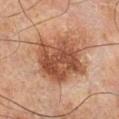Assessment:
Recorded during total-body skin imaging; not selected for excision or biopsy.
Background:
This image is a 15 mm lesion crop taken from a total-body photograph. A male patient, roughly 65 years of age. From the right lower leg.